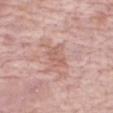The lesion was photographed on a routine skin check and not biopsied; there is no pathology result. Approximately 3 mm at its widest. A 15 mm crop from a total-body photograph taken for skin-cancer surveillance. On the right forearm. The lesion-visualizer software estimated a color-variation rating of about 1.5/10 and peripheral color asymmetry of about 0.5. And it measured a classifier nevus-likeness of about 0/100 and a lesion-detection confidence of about 95/100. A male patient approximately 75 years of age. Imaged with white-light lighting.